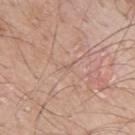biopsy status: no biopsy performed (imaged during a skin exam)
illumination: white-light
patient: male, aged 58 to 62
body site: the left upper arm
acquisition: ~15 mm crop, total-body skin-cancer survey
lesion diameter: ~1 mm (longest diameter)
TBP lesion metrics: a lesion area of about 1 mm², a shape eccentricity near 0.85, and two-axis asymmetry of about 0.3; a lesion–skin lightness drop of about 5 and a normalized lesion–skin contrast near 3.5